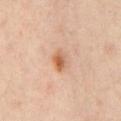* workup · imaged on a skin check; not biopsied
* acquisition · ~15 mm crop, total-body skin-cancer survey
* body site · the chest
* patient · aged approximately 55